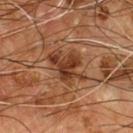This lesion was catalogued during total-body skin photography and was not selected for biopsy. A roughly 15 mm field-of-view crop from a total-body skin photograph. A male subject roughly 55 years of age. The lesion is located on the front of the torso. Captured under cross-polarized illumination.